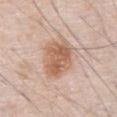  biopsy_status: not biopsied; imaged during a skin examination
  site: abdomen
  patient:
    sex: male
    age_approx: 80
  image:
    source: total-body photography crop
    field_of_view_mm: 15